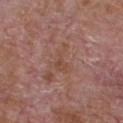* follow-up · total-body-photography surveillance lesion; no biopsy
* diameter · ~3.5 mm (longest diameter)
* lighting · white-light illumination
* acquisition · ~15 mm tile from a whole-body skin photo
* subject · male, aged 63–67
* anatomic site · the front of the torso
* automated lesion analysis · a classifier nevus-likeness of about 0/100 and a lesion-detection confidence of about 95/100